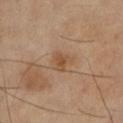  biopsy_status: not biopsied; imaged during a skin examination
  site: leg
  patient:
    sex: female
    age_approx: 70
  image:
    source: total-body photography crop
    field_of_view_mm: 15